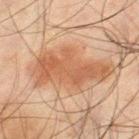follow-up: imaged on a skin check; not biopsied | site: the left thigh | illumination: cross-polarized | automated lesion analysis: a lesion area of about 24 mm², an outline eccentricity of about 0.9 (0 = round, 1 = elongated), and a symmetry-axis asymmetry near 0.4; a classifier nevus-likeness of about 30/100 | image: ~15 mm tile from a whole-body skin photo | size: ≈8.5 mm | subject: male, approximately 45 years of age.